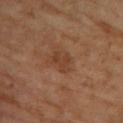Notes:
* follow-up: total-body-photography surveillance lesion; no biopsy
* tile lighting: cross-polarized
* imaging modality: 15 mm crop, total-body photography
* automated lesion analysis: a shape eccentricity near 0.7 and a symmetry-axis asymmetry near 0.3; a border-irregularity index near 3.5/10 and internal color variation of about 2 on a 0–10 scale
* site: the upper back
* subject: female, about 65 years old